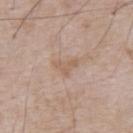<record>
  <biopsy_status>not biopsied; imaged during a skin examination</biopsy_status>
  <lighting>white-light</lighting>
  <automated_metrics>
    <eccentricity>0.75</eccentricity>
    <shape_asymmetry>0.55</shape_asymmetry>
    <color_variation_0_10>1.0</color_variation_0_10>
    <peripheral_color_asymmetry>0.5</peripheral_color_asymmetry>
  </automated_metrics>
  <patient>
    <sex>male</sex>
    <age_approx>80</age_approx>
  </patient>
  <site>chest</site>
  <lesion_size>
    <long_diameter_mm_approx>3.0</long_diameter_mm_approx>
  </lesion_size>
  <image>
    <source>total-body photography crop</source>
    <field_of_view_mm>15</field_of_view_mm>
  </image>
</record>